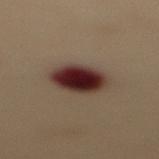notes — catalogued during a skin exam; not biopsied